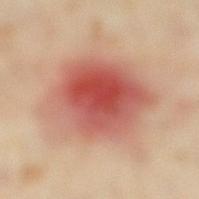<tbp_lesion>
<biopsy_status>not biopsied; imaged during a skin examination</biopsy_status>
<site>right lower leg</site>
<automated_metrics>
  <area_mm2_approx>45.0</area_mm2_approx>
  <eccentricity>0.6</eccentricity>
  <shape_asymmetry>0.2</shape_asymmetry>
  <cielab_L>54</cielab_L>
  <cielab_a>27</cielab_a>
  <cielab_b>28</cielab_b>
  <vs_skin_darker_L>13.0</vs_skin_darker_L>
  <vs_skin_contrast_norm>9.0</vs_skin_contrast_norm>
  <lesion_detection_confidence_0_100>100</lesion_detection_confidence_0_100>
</automated_metrics>
<lesion_size>
  <long_diameter_mm_approx>9.0</long_diameter_mm_approx>
</lesion_size>
<image>
  <source>total-body photography crop</source>
  <field_of_view_mm>15</field_of_view_mm>
</image>
<lighting>cross-polarized</lighting>
<patient>
  <sex>female</sex>
  <age_approx>35</age_approx>
</patient>
</tbp_lesion>